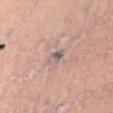biopsy status: no biopsy performed (imaged during a skin exam) | location: the front of the torso | lesion size: ≈3 mm | illumination: white-light illumination | patient: male, in their 50s | image source: total-body-photography crop, ~15 mm field of view.